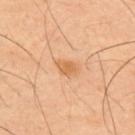Captured during whole-body skin photography for melanoma surveillance; the lesion was not biopsied. A male patient, roughly 60 years of age. The recorded lesion diameter is about 2.5 mm. This image is a 15 mm lesion crop taken from a total-body photograph. On the upper back. The tile uses cross-polarized illumination.